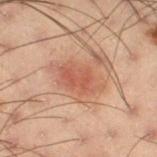<case>
  <biopsy_status>not biopsied; imaged during a skin examination</biopsy_status>
  <lesion_size>
    <long_diameter_mm_approx>5.5</long_diameter_mm_approx>
  </lesion_size>
  <image>
    <source>total-body photography crop</source>
    <field_of_view_mm>15</field_of_view_mm>
  </image>
  <patient>
    <sex>male</sex>
    <age_approx>55</age_approx>
  </patient>
  <site>right thigh</site>
</case>